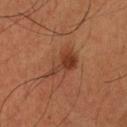On the head or neck. A male subject aged approximately 35. Cropped from a total-body skin-imaging series; the visible field is about 15 mm. The total-body-photography lesion software estimated a footprint of about 8.5 mm², an eccentricity of roughly 0.8, and two-axis asymmetry of about 0.45. The software also gave a border-irregularity rating of about 5/10, internal color variation of about 5 on a 0–10 scale, and peripheral color asymmetry of about 2. Longest diameter approximately 4.5 mm. Captured under cross-polarized illumination.